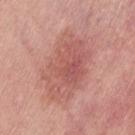Q: How large is the lesion?
A: about 8 mm
Q: Lesion location?
A: the left thigh
Q: What did automated image analysis measure?
A: a footprint of about 31 mm², an outline eccentricity of about 0.8 (0 = round, 1 = elongated), and a shape-asymmetry score of about 0.2 (0 = symmetric); lesion-presence confidence of about 100/100
Q: How was this image acquired?
A: 15 mm crop, total-body photography
Q: How was the tile lit?
A: white-light
Q: Who is the patient?
A: female, in their 40s